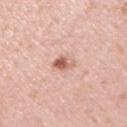Impression:
This lesion was catalogued during total-body skin photography and was not selected for biopsy.
Image and clinical context:
Captured under white-light illumination. A lesion tile, about 15 mm wide, cut from a 3D total-body photograph. A male patient aged 48–52. Located on the right upper arm.